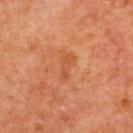<record>
<automated_metrics>
  <cielab_L>54</cielab_L>
  <cielab_a>30</cielab_a>
  <cielab_b>42</cielab_b>
  <vs_skin_darker_L>6.0</vs_skin_darker_L>
  <vs_skin_contrast_norm>5.0</vs_skin_contrast_norm>
  <color_variation_0_10>1.0</color_variation_0_10>
  <peripheral_color_asymmetry>0.5</peripheral_color_asymmetry>
  <nevus_likeness_0_100>0</nevus_likeness_0_100>
  <lesion_detection_confidence_0_100>100</lesion_detection_confidence_0_100>
</automated_metrics>
<image>
  <source>total-body photography crop</source>
  <field_of_view_mm>15</field_of_view_mm>
</image>
<lesion_size>
  <long_diameter_mm_approx>3.0</long_diameter_mm_approx>
</lesion_size>
<patient>
  <sex>female</sex>
  <age_approx>40</age_approx>
</patient>
<lighting>cross-polarized</lighting>
<site>upper back</site>
</record>